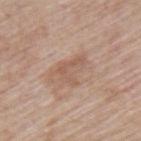The lesion was photographed on a routine skin check and not biopsied; there is no pathology result. The subject is a male aged approximately 60. Automated image analysis of the tile measured a footprint of about 7.5 mm², an outline eccentricity of about 0.7 (0 = round, 1 = elongated), and two-axis asymmetry of about 0.55. It also reported a border-irregularity index near 6/10 and peripheral color asymmetry of about 1. On the upper back. The lesion's longest dimension is about 4 mm. A region of skin cropped from a whole-body photographic capture, roughly 15 mm wide.